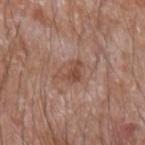<tbp_lesion>
  <biopsy_status>not biopsied; imaged during a skin examination</biopsy_status>
  <patient>
    <sex>male</sex>
    <age_approx>60</age_approx>
  </patient>
  <site>left forearm</site>
  <automated_metrics>
    <border_irregularity_0_10>6.0</border_irregularity_0_10>
    <color_variation_0_10>2.0</color_variation_0_10>
    <nevus_likeness_0_100>0</nevus_likeness_0_100>
  </automated_metrics>
  <lesion_size>
    <long_diameter_mm_approx>3.0</long_diameter_mm_approx>
  </lesion_size>
  <image>
    <source>total-body photography crop</source>
    <field_of_view_mm>15</field_of_view_mm>
  </image>
</tbp_lesion>